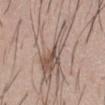| key | value |
|---|---|
| workup | catalogued during a skin exam; not biopsied |
| automated metrics | a lesion area of about 25 mm² and an eccentricity of roughly 0.8; a lesion color around L≈57 a*≈14 b*≈23 in CIELAB, a lesion–skin lightness drop of about 9, and a lesion-to-skin contrast of about 6 (normalized; higher = more distinct); lesion-presence confidence of about 50/100 |
| diameter | ~9 mm (longest diameter) |
| acquisition | ~15 mm tile from a whole-body skin photo |
| subject | male, aged 28 to 32 |
| illumination | white-light illumination |
| site | the abdomen |